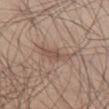notes = catalogued during a skin exam; not biopsied
site = the leg
image source = ~15 mm crop, total-body skin-cancer survey
automated lesion analysis = an area of roughly 5 mm² and a symmetry-axis asymmetry near 0.4
patient = male, about 45 years old